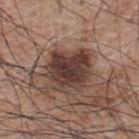{"image": {"source": "total-body photography crop", "field_of_view_mm": 15}, "lighting": "white-light", "lesion_size": {"long_diameter_mm_approx": 5.0}, "automated_metrics": {"area_mm2_approx": 19.0, "shape_asymmetry": 0.2}, "patient": {"sex": "male", "age_approx": 70}, "site": "upper back"}